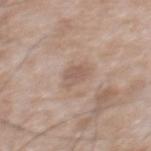illumination: white-light | subject: male, approximately 50 years of age | site: the mid back | diameter: ~3 mm (longest diameter) | image source: total-body-photography crop, ~15 mm field of view.